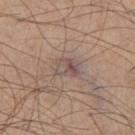The lesion was tiled from a total-body skin photograph and was not biopsied. This is a white-light tile. Automated image analysis of the tile measured a footprint of about 5.5 mm², an outline eccentricity of about 0.7 (0 = round, 1 = elongated), and a symmetry-axis asymmetry near 0.25. The analysis additionally found an average lesion color of about L≈51 a*≈15 b*≈20 (CIELAB). The analysis additionally found a border-irregularity index near 2.5/10, a within-lesion color-variation index near 8/10, and radial color variation of about 3. The software also gave a lesion-detection confidence of about 60/100. A male patient aged approximately 65. A 15 mm crop from a total-body photograph taken for skin-cancer surveillance. Approximately 3 mm at its widest. From the left thigh.